A 15 mm close-up tile from a total-body photography series done for melanoma screening · located on the left lower leg · the lesion's longest dimension is about 7 mm · a male subject about 65 years old · this is a cross-polarized tile — 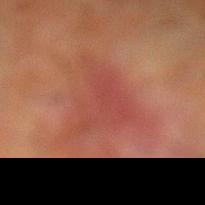Biopsy histopathology demonstrated a superficial basal cell carcinoma.